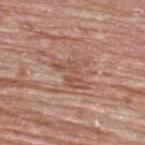Q: Is there a histopathology result?
A: total-body-photography surveillance lesion; no biopsy
Q: How was this image acquired?
A: total-body-photography crop, ~15 mm field of view
Q: How large is the lesion?
A: ~4 mm (longest diameter)
Q: Patient demographics?
A: male, roughly 65 years of age
Q: What is the anatomic site?
A: the upper back
Q: What lighting was used for the tile?
A: white-light illumination